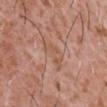This lesion was catalogued during total-body skin photography and was not selected for biopsy. A region of skin cropped from a whole-body photographic capture, roughly 15 mm wide. On the chest. A male subject, aged approximately 45. Measured at roughly 4.5 mm in maximum diameter.